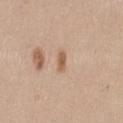Case summary:
• follow-up: total-body-photography surveillance lesion; no biopsy
• subject: female, approximately 40 years of age
• automated lesion analysis: a border-irregularity index near 2.5/10, a color-variation rating of about 0.5/10, and a peripheral color-asymmetry measure near 0
• location: the left thigh
• image source: total-body-photography crop, ~15 mm field of view
• lighting: white-light
• lesion size: ≈2.5 mm